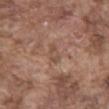Q: Is there a histopathology result?
A: catalogued during a skin exam; not biopsied
Q: What is the imaging modality?
A: ~15 mm tile from a whole-body skin photo
Q: What is the anatomic site?
A: the chest
Q: Patient demographics?
A: male, aged around 75